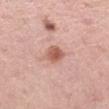{"biopsy_status": "not biopsied; imaged during a skin examination", "site": "left thigh", "patient": {"sex": "female", "age_approx": 45}, "image": {"source": "total-body photography crop", "field_of_view_mm": 15}}